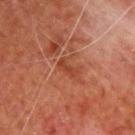follow-up = catalogued during a skin exam; not biopsied
diameter = ~3 mm (longest diameter)
patient = male, about 65 years old
anatomic site = the upper back
image = 15 mm crop, total-body photography